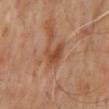- follow-up · catalogued during a skin exam; not biopsied
- patient · male, roughly 65 years of age
- anatomic site · the back
- acquisition · ~15 mm crop, total-body skin-cancer survey
- lesion diameter · ~3 mm (longest diameter)